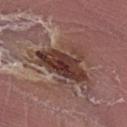This lesion was catalogued during total-body skin photography and was not selected for biopsy. The subject is a male aged 63 to 67. On the arm. A close-up tile cropped from a whole-body skin photograph, about 15 mm across. The total-body-photography lesion software estimated a footprint of about 16 mm² and an eccentricity of roughly 0.8. The analysis additionally found a border-irregularity index near 6/10 and radial color variation of about 2. The analysis additionally found an automated nevus-likeness rating near 0 out of 100.